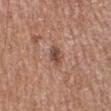* workup — no biopsy performed (imaged during a skin exam)
* subject — male, approximately 70 years of age
* body site — the right lower leg
* diameter — about 2.5 mm
* image source — ~15 mm tile from a whole-body skin photo
* tile lighting — white-light